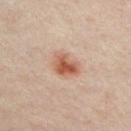{"biopsy_status": "not biopsied; imaged during a skin examination", "image": {"source": "total-body photography crop", "field_of_view_mm": 15}, "automated_metrics": {"cielab_L": 55, "cielab_a": 22, "cielab_b": 30, "vs_skin_darker_L": 12.0}, "lighting": "cross-polarized", "patient": {"sex": "male", "age_approx": 35}, "site": "chest"}